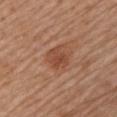{
  "biopsy_status": "not biopsied; imaged during a skin examination",
  "patient": {
    "sex": "male",
    "age_approx": 65
  },
  "site": "left upper arm",
  "image": {
    "source": "total-body photography crop",
    "field_of_view_mm": 15
  },
  "lesion_size": {
    "long_diameter_mm_approx": 3.0
  },
  "automated_metrics": {
    "border_irregularity_0_10": 1.5,
    "peripheral_color_asymmetry": 1.0
  },
  "lighting": "white-light"
}